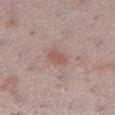The lesion was photographed on a routine skin check and not biopsied; there is no pathology result.